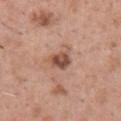The lesion was photographed on a routine skin check and not biopsied; there is no pathology result. A male subject in their mid-40s. Automated image analysis of the tile measured an average lesion color of about L≈51 a*≈22 b*≈28 (CIELAB) and a lesion–skin lightness drop of about 13. The analysis additionally found internal color variation of about 5 on a 0–10 scale. And it measured a nevus-likeness score of about 50/100 and a lesion-detection confidence of about 100/100. The tile uses white-light illumination. Located on the chest. A 15 mm crop from a total-body photograph taken for skin-cancer surveillance. The recorded lesion diameter is about 3.5 mm.